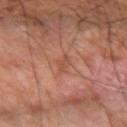A roughly 15 mm field-of-view crop from a total-body skin photograph. A male subject aged 58–62. The tile uses cross-polarized illumination. An algorithmic analysis of the crop reported an outline eccentricity of about 0.9 (0 = round, 1 = elongated). The software also gave an automated nevus-likeness rating near 0 out of 100 and lesion-presence confidence of about 90/100. Longest diameter approximately 3 mm. The lesion is located on the left forearm.